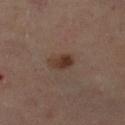Part of a total-body skin-imaging series; this lesion was reviewed on a skin check and was not flagged for biopsy. From the right lower leg. The subject is a female aged approximately 60. The tile uses cross-polarized illumination. A roughly 15 mm field-of-view crop from a total-body skin photograph. Measured at roughly 3 mm in maximum diameter.